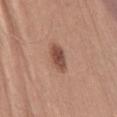No biopsy was performed on this lesion — it was imaged during a full skin examination and was not determined to be concerning.
Cropped from a total-body skin-imaging series; the visible field is about 15 mm.
A male subject, aged 53 to 57.
Located on the abdomen.
The lesion-visualizer software estimated an average lesion color of about L≈50 a*≈22 b*≈28 (CIELAB) and about 13 CIELAB-L* units darker than the surrounding skin.
The tile uses white-light illumination.
Longest diameter approximately 4 mm.